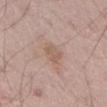Assessment:
Part of a total-body skin-imaging series; this lesion was reviewed on a skin check and was not flagged for biopsy.
Context:
An algorithmic analysis of the crop reported a border-irregularity index near 3.5/10, internal color variation of about 2 on a 0–10 scale, and radial color variation of about 0.5. Located on the abdomen. The subject is a male about 65 years old. The tile uses white-light illumination. Cropped from a total-body skin-imaging series; the visible field is about 15 mm.